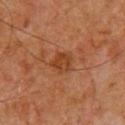workup = no biopsy performed (imaged during a skin exam)
acquisition = ~15 mm crop, total-body skin-cancer survey
lighting = cross-polarized
subject = male, aged 58–62
automated lesion analysis = an average lesion color of about L≈38 a*≈24 b*≈34 (CIELAB), about 7 CIELAB-L* units darker than the surrounding skin, and a normalized lesion–skin contrast near 7; a classifier nevus-likeness of about 5/100 and a detector confidence of about 100 out of 100 that the crop contains a lesion
lesion size = ~3 mm (longest diameter)
location = the upper back